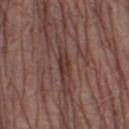The lesion was photographed on a routine skin check and not biopsied; there is no pathology result.
The subject is a male in their mid- to late 60s.
About 2.5 mm across.
Cropped from a whole-body photographic skin survey; the tile spans about 15 mm.
On the left thigh.
This is a white-light tile.
The total-body-photography lesion software estimated a lesion area of about 3.5 mm², a shape eccentricity near 0.85, and two-axis asymmetry of about 0.3. The analysis additionally found an average lesion color of about L≈34 a*≈20 b*≈21 (CIELAB), a lesion–skin lightness drop of about 8, and a normalized border contrast of about 7.5. It also reported a border-irregularity index near 3/10 and a within-lesion color-variation index near 1/10.